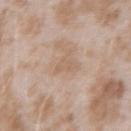biopsy status: no biopsy performed (imaged during a skin exam)
lesion size: ≈3 mm
subject: female, approximately 25 years of age
lighting: white-light illumination
anatomic site: the arm
imaging modality: total-body-photography crop, ~15 mm field of view
automated metrics: an area of roughly 3 mm² and a shape-asymmetry score of about 0.4 (0 = symmetric); a lesion color around L≈61 a*≈16 b*≈30 in CIELAB, roughly 6 lightness units darker than nearby skin, and a lesion-to-skin contrast of about 4.5 (normalized; higher = more distinct); a within-lesion color-variation index near 1.5/10 and radial color variation of about 0.5; a nevus-likeness score of about 0/100